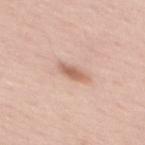Recorded during total-body skin imaging; not selected for excision or biopsy. The lesion's longest dimension is about 3 mm. Automated tile analysis of the lesion measured a lesion area of about 4 mm², an eccentricity of roughly 0.9, and a symmetry-axis asymmetry near 0.25. It also reported a mean CIELAB color near L≈62 a*≈21 b*≈29. The software also gave a border-irregularity rating of about 3/10, internal color variation of about 2 on a 0–10 scale, and radial color variation of about 0.5. And it measured an automated nevus-likeness rating near 90 out of 100 and lesion-presence confidence of about 100/100. Imaged with white-light lighting. The subject is a female aged 33 to 37. On the mid back. Cropped from a total-body skin-imaging series; the visible field is about 15 mm.